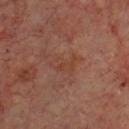Q: What is the anatomic site?
A: the chest
Q: Illumination type?
A: cross-polarized illumination
Q: What is the imaging modality?
A: ~15 mm crop, total-body skin-cancer survey
Q: Patient demographics?
A: male, roughly 70 years of age
Q: Lesion size?
A: ≈3.5 mm